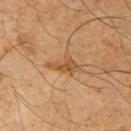follow-up=no biopsy performed (imaged during a skin exam) | image=~15 mm crop, total-body skin-cancer survey | location=the right upper arm | illumination=cross-polarized illumination | subject=male, roughly 65 years of age | diameter=≈4 mm.